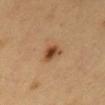Assessment: Part of a total-body skin-imaging series; this lesion was reviewed on a skin check and was not flagged for biopsy. Context: The patient is a male aged approximately 60. Cropped from a total-body skin-imaging series; the visible field is about 15 mm. Automated image analysis of the tile measured roughly 13 lightness units darker than nearby skin and a normalized lesion–skin contrast near 10. And it measured a within-lesion color-variation index near 6/10 and peripheral color asymmetry of about 2. The analysis additionally found a nevus-likeness score of about 95/100 and a lesion-detection confidence of about 100/100. Located on the abdomen. This is a cross-polarized tile. About 2.5 mm across.